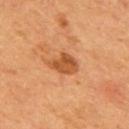Impression:
Imaged during a routine full-body skin examination; the lesion was not biopsied and no histopathology is available.
Acquisition and patient details:
The lesion is located on the back. A male patient aged 53–57. A region of skin cropped from a whole-body photographic capture, roughly 15 mm wide.